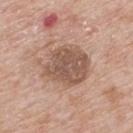Clinical impression: This lesion was catalogued during total-body skin photography and was not selected for biopsy. Acquisition and patient details: Captured under white-light illumination. Measured at roughly 6 mm in maximum diameter. A male patient approximately 65 years of age. The lesion is located on the back. A close-up tile cropped from a whole-body skin photograph, about 15 mm across.